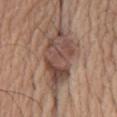Assessment: Recorded during total-body skin imaging; not selected for excision or biopsy. Image and clinical context: An algorithmic analysis of the crop reported an area of roughly 19 mm², an eccentricity of roughly 0.9, and a shape-asymmetry score of about 0.3 (0 = symmetric). It also reported a mean CIELAB color near L≈47 a*≈18 b*≈24, a lesion–skin lightness drop of about 12, and a normalized border contrast of about 9. The lesion's longest dimension is about 8.5 mm. The lesion is on the front of the torso. A close-up tile cropped from a whole-body skin photograph, about 15 mm across. The patient is a female aged 73 to 77.